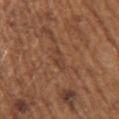Notes:
– notes — no biopsy performed (imaged during a skin exam)
– location — the left upper arm
– TBP lesion metrics — a border-irregularity index near 4.5/10, internal color variation of about 1.5 on a 0–10 scale, and peripheral color asymmetry of about 0.5; a classifier nevus-likeness of about 0/100 and a detector confidence of about 70 out of 100 that the crop contains a lesion
– illumination — white-light illumination
– diameter — about 2.5 mm
– acquisition — 15 mm crop, total-body photography
– patient — female, approximately 75 years of age